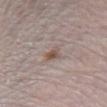follow-up = catalogued during a skin exam; not biopsied | site = the right lower leg | lighting = white-light illumination | subject = female, aged 28–32 | imaging modality = total-body-photography crop, ~15 mm field of view | size = ≈3.5 mm.